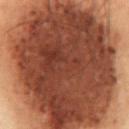<tbp_lesion>
  <biopsy_status>not biopsied; imaged during a skin examination</biopsy_status>
</tbp_lesion>